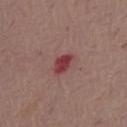Captured during whole-body skin photography for melanoma surveillance; the lesion was not biopsied. The tile uses white-light illumination. A 15 mm close-up tile from a total-body photography series done for melanoma screening. The subject is a male aged around 70. The lesion's longest dimension is about 3 mm. The lesion is on the front of the torso. An algorithmic analysis of the crop reported an average lesion color of about L≈40 a*≈29 b*≈19 (CIELAB) and about 11 CIELAB-L* units darker than the surrounding skin. And it measured a classifier nevus-likeness of about 0/100.